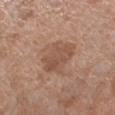Q: Was a biopsy performed?
A: imaged on a skin check; not biopsied
Q: Where on the body is the lesion?
A: the right forearm
Q: What kind of image is this?
A: 15 mm crop, total-body photography
Q: What are the patient's age and sex?
A: female, about 50 years old